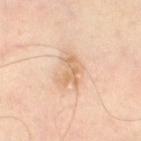Impression: Imaged during a routine full-body skin examination; the lesion was not biopsied and no histopathology is available. Background: The subject is a male about 50 years old. A 15 mm crop from a total-body photograph taken for skin-cancer surveillance. The recorded lesion diameter is about 3.5 mm. The lesion-visualizer software estimated an average lesion color of about L≈68 a*≈18 b*≈35 (CIELAB), about 9 CIELAB-L* units darker than the surrounding skin, and a lesion-to-skin contrast of about 6 (normalized; higher = more distinct). The analysis additionally found an automated nevus-likeness rating near 0 out of 100 and a lesion-detection confidence of about 100/100. From the left thigh.